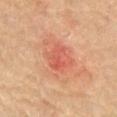This lesion was catalogued during total-body skin photography and was not selected for biopsy. A roughly 15 mm field-of-view crop from a total-body skin photograph. Automated tile analysis of the lesion measured an area of roughly 4 mm² and an eccentricity of roughly 0.85. And it measured border irregularity of about 5 on a 0–10 scale, a color-variation rating of about 1/10, and radial color variation of about 0. A male subject, in their mid-70s. Approximately 3 mm at its widest. Located on the abdomen. This is a cross-polarized tile.